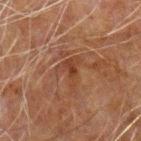Assessment: The lesion was tiled from a total-body skin photograph and was not biopsied.